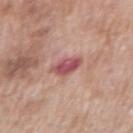{"biopsy_status": "not biopsied; imaged during a skin examination", "image": {"source": "total-body photography crop", "field_of_view_mm": 15}, "site": "right upper arm", "patient": {"sex": "male", "age_approx": 70}, "lesion_size": {"long_diameter_mm_approx": 3.5}, "automated_metrics": {"eccentricity": 0.75, "shape_asymmetry": 0.2, "cielab_L": 52, "cielab_a": 29, "cielab_b": 21, "vs_skin_darker_L": 13.0, "nevus_likeness_0_100": 0, "lesion_detection_confidence_0_100": 100}, "lighting": "white-light"}